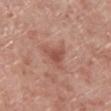| key | value |
|---|---|
| follow-up | no biopsy performed (imaged during a skin exam) |
| anatomic site | the right lower leg |
| acquisition | ~15 mm crop, total-body skin-cancer survey |
| subject | male, aged around 70 |